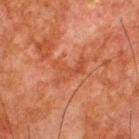size: ≈4.5 mm
image source: 15 mm crop, total-body photography
location: the upper back
illumination: cross-polarized illumination
patient: male, roughly 65 years of age